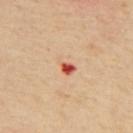<case>
<biopsy_status>not biopsied; imaged during a skin examination</biopsy_status>
<patient>
  <sex>male</sex>
  <age_approx>55</age_approx>
</patient>
<site>mid back</site>
<lighting>cross-polarized</lighting>
<image>
  <source>total-body photography crop</source>
  <field_of_view_mm>15</field_of_view_mm>
</image>
<lesion_size>
  <long_diameter_mm_approx>3.0</long_diameter_mm_approx>
</lesion_size>
</case>